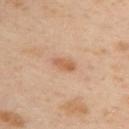image source: 15 mm crop, total-body photography
automated metrics: a lesion area of about 3.5 mm² and an outline eccentricity of about 0.9 (0 = round, 1 = elongated); an automated nevus-likeness rating near 55 out of 100 and a lesion-detection confidence of about 100/100
diameter: about 3 mm
patient: female, approximately 40 years of age
anatomic site: the upper back